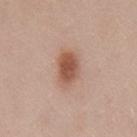{"biopsy_status": "not biopsied; imaged during a skin examination", "image": {"source": "total-body photography crop", "field_of_view_mm": 15}, "site": "chest", "lighting": "white-light", "lesion_size": {"long_diameter_mm_approx": 4.0}, "automated_metrics": {"eccentricity": 0.8, "cielab_L": 54, "cielab_a": 22, "cielab_b": 29, "vs_skin_darker_L": 13.0, "vs_skin_contrast_norm": 9.0, "border_irregularity_0_10": 2.0, "nevus_likeness_0_100": 100, "lesion_detection_confidence_0_100": 100}, "patient": {"sex": "male", "age_approx": 55}}